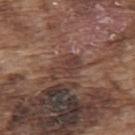  patient:
    sex: male
    age_approx: 75
  lighting: white-light
  lesion_size:
    long_diameter_mm_approx: 4.0
  automated_metrics:
    eccentricity: 0.9
    shape_asymmetry: 0.45
    border_irregularity_0_10: 6.5
    peripheral_color_asymmetry: 0.5
  site: upper back
  image:
    source: total-body photography crop
    field_of_view_mm: 15